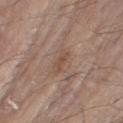| feature | finding |
|---|---|
| notes | total-body-photography surveillance lesion; no biopsy |
| patient | male, about 80 years old |
| image | 15 mm crop, total-body photography |
| location | the right thigh |